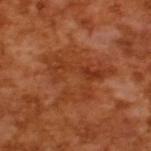Assessment: Recorded during total-body skin imaging; not selected for excision or biopsy. Background: This is a cross-polarized tile. The subject is a male roughly 65 years of age. A 15 mm close-up extracted from a 3D total-body photography capture. About 7 mm across. The total-body-photography lesion software estimated an area of roughly 22 mm². The analysis additionally found a nevus-likeness score of about 0/100 and lesion-presence confidence of about 90/100.